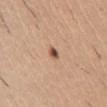<record>
  <biopsy_status>not biopsied; imaged during a skin examination</biopsy_status>
  <patient>
    <sex>male</sex>
    <age_approx>40</age_approx>
  </patient>
  <automated_metrics>
    <color_variation_0_10>2.0</color_variation_0_10>
    <peripheral_color_asymmetry>0.5</peripheral_color_asymmetry>
    <nevus_likeness_0_100>95</nevus_likeness_0_100>
    <lesion_detection_confidence_0_100>100</lesion_detection_confidence_0_100>
  </automated_metrics>
  <image>
    <source>total-body photography crop</source>
    <field_of_view_mm>15</field_of_view_mm>
  </image>
  <site>front of the torso</site>
  <lighting>white-light</lighting>
  <lesion_size>
    <long_diameter_mm_approx>1.5</long_diameter_mm_approx>
  </lesion_size>
</record>